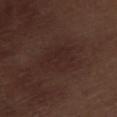| feature | finding |
|---|---|
| follow-up | imaged on a skin check; not biopsied |
| automated lesion analysis | about 4 CIELAB-L* units darker than the surrounding skin and a normalized border contrast of about 4.5; border irregularity of about 3.5 on a 0–10 scale, a color-variation rating of about 2/10, and peripheral color asymmetry of about 0.5; a nevus-likeness score of about 0/100 and a lesion-detection confidence of about 100/100 |
| tile lighting | white-light illumination |
| imaging modality | ~15 mm crop, total-body skin-cancer survey |
| anatomic site | the leg |
| subject | male, aged 68 to 72 |
| diameter | about 4.5 mm |